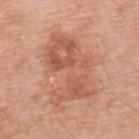The lesion was tiled from a total-body skin photograph and was not biopsied. Captured under white-light illumination. A close-up tile cropped from a whole-body skin photograph, about 15 mm across. A male subject aged around 60. The lesion-visualizer software estimated a shape eccentricity near 0.8 and a shape-asymmetry score of about 0.45 (0 = symmetric). The software also gave border irregularity of about 7.5 on a 0–10 scale, a color-variation rating of about 6/10, and a peripheral color-asymmetry measure near 2.5. It also reported a nevus-likeness score of about 0/100 and a lesion-detection confidence of about 100/100. Located on the upper back. Measured at roughly 8 mm in maximum diameter.